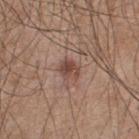Part of a total-body skin-imaging series; this lesion was reviewed on a skin check and was not flagged for biopsy.
Located on the upper back.
About 2.5 mm across.
A 15 mm crop from a total-body photograph taken for skin-cancer surveillance.
A male subject, approximately 45 years of age.
This is a white-light tile.
Automated tile analysis of the lesion measured a footprint of about 5 mm², a shape eccentricity near 0.55, and a symmetry-axis asymmetry near 0.2. The software also gave a border-irregularity index near 2/10 and a color-variation rating of about 5/10. And it measured a classifier nevus-likeness of about 85/100 and a detector confidence of about 100 out of 100 that the crop contains a lesion.